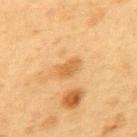Notes:
- notes · imaged on a skin check; not biopsied
- body site · the upper back
- illumination · cross-polarized
- lesion diameter · ≈2.5 mm
- image · 15 mm crop, total-body photography
- patient · male, aged 53–57